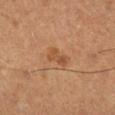<tbp_lesion>
  <biopsy_status>not biopsied; imaged during a skin examination</biopsy_status>
  <lesion_size>
    <long_diameter_mm_approx>2.5</long_diameter_mm_approx>
  </lesion_size>
  <image>
    <source>total-body photography crop</source>
    <field_of_view_mm>15</field_of_view_mm>
  </image>
  <patient>
    <sex>male</sex>
    <age_approx>55</age_approx>
  </patient>
  <lighting>cross-polarized</lighting>
  <site>right lower leg</site>
</tbp_lesion>